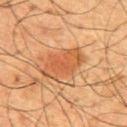Captured during whole-body skin photography for melanoma surveillance; the lesion was not biopsied.
A male patient approximately 60 years of age.
This image is a 15 mm lesion crop taken from a total-body photograph.
Automated image analysis of the tile measured a classifier nevus-likeness of about 95/100 and lesion-presence confidence of about 100/100.
The lesion is located on the upper back.